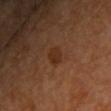<lesion>
  <biopsy_status>not biopsied; imaged during a skin examination</biopsy_status>
  <lighting>cross-polarized</lighting>
  <image>
    <source>total-body photography crop</source>
    <field_of_view_mm>15</field_of_view_mm>
  </image>
  <automated_metrics>
    <area_mm2_approx>4.0</area_mm2_approx>
    <eccentricity>0.65</eccentricity>
    <shape_asymmetry>0.2</shape_asymmetry>
  </automated_metrics>
  <patient>
    <sex>female</sex>
    <age_approx>60</age_approx>
  </patient>
  <site>upper back</site>
  <lesion_size>
    <long_diameter_mm_approx>2.5</long_diameter_mm_approx>
  </lesion_size>
</lesion>